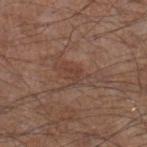{"biopsy_status": "not biopsied; imaged during a skin examination", "automated_metrics": {"cielab_L": 40, "cielab_a": 20, "cielab_b": 25, "vs_skin_contrast_norm": 5.5, "border_irregularity_0_10": 6.5, "color_variation_0_10": 0.5, "peripheral_color_asymmetry": 0.0, "nevus_likeness_0_100": 0, "lesion_detection_confidence_0_100": 95}, "image": {"source": "total-body photography crop", "field_of_view_mm": 15}, "patient": {"sex": "male", "age_approx": 55}, "site": "right lower leg", "lesion_size": {"long_diameter_mm_approx": 2.5}}